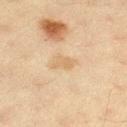Clinical impression: No biopsy was performed on this lesion — it was imaged during a full skin examination and was not determined to be concerning. Context: The lesion's longest dimension is about 2.5 mm. The lesion is on the left thigh. A female patient aged 38–42. A roughly 15 mm field-of-view crop from a total-body skin photograph.